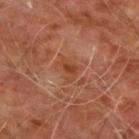Measured at roughly 3 mm in maximum diameter. A male patient about 60 years old. The lesion is located on the chest. Automated image analysis of the tile measured a lesion area of about 3.5 mm², an outline eccentricity of about 0.75 (0 = round, 1 = elongated), and a symmetry-axis asymmetry near 0.55. And it measured border irregularity of about 5.5 on a 0–10 scale and a color-variation rating of about 1/10. The software also gave an automated nevus-likeness rating near 0 out of 100 and a detector confidence of about 100 out of 100 that the crop contains a lesion. A roughly 15 mm field-of-view crop from a total-body skin photograph.